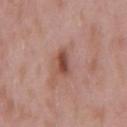Impression:
Part of a total-body skin-imaging series; this lesion was reviewed on a skin check and was not flagged for biopsy.
Acquisition and patient details:
A male subject, roughly 55 years of age. This is a white-light tile. An algorithmic analysis of the crop reported a footprint of about 4.5 mm², a shape eccentricity near 0.8, and a symmetry-axis asymmetry near 0.3. And it measured a classifier nevus-likeness of about 80/100. A 15 mm close-up extracted from a 3D total-body photography capture. From the back.